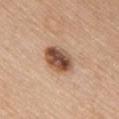Part of a total-body skin-imaging series; this lesion was reviewed on a skin check and was not flagged for biopsy. The lesion-visualizer software estimated an area of roughly 10 mm² and two-axis asymmetry of about 0.1. And it measured a lesion color around L≈51 a*≈21 b*≈31 in CIELAB. The analysis additionally found a border-irregularity rating of about 1/10, internal color variation of about 8.5 on a 0–10 scale, and radial color variation of about 3. It also reported a nevus-likeness score of about 90/100 and lesion-presence confidence of about 100/100. From the chest. Captured under white-light illumination. A 15 mm close-up extracted from a 3D total-body photography capture. The patient is a male aged 38–42.